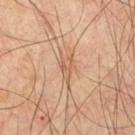{"biopsy_status": "not biopsied; imaged during a skin examination", "lighting": "cross-polarized", "automated_metrics": {"area_mm2_approx": 3.5, "eccentricity": 0.8, "shape_asymmetry": 0.4, "cielab_L": 58, "cielab_a": 20, "cielab_b": 33, "vs_skin_darker_L": 8.0, "color_variation_0_10": 2.5, "peripheral_color_asymmetry": 1.0}, "site": "chest", "image": {"source": "total-body photography crop", "field_of_view_mm": 15}, "patient": {"sex": "male", "age_approx": 60}, "lesion_size": {"long_diameter_mm_approx": 3.0}}